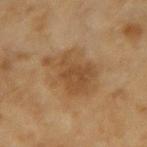Notes:
• follow-up · catalogued during a skin exam; not biopsied
• site · the left forearm
• patient · female, aged around 60
• lesion diameter · about 7 mm
• illumination · cross-polarized
• image source · ~15 mm tile from a whole-body skin photo